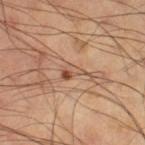Case summary:
- biopsy status · imaged on a skin check; not biopsied
- anatomic site · the right thigh
- diameter · about 3 mm
- subject · male, aged approximately 60
- image · total-body-photography crop, ~15 mm field of view
- illumination · cross-polarized illumination